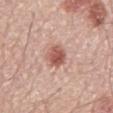Impression:
No biopsy was performed on this lesion — it was imaged during a full skin examination and was not determined to be concerning.
Clinical summary:
On the mid back. This is a white-light tile. The recorded lesion diameter is about 3 mm. Cropped from a whole-body photographic skin survey; the tile spans about 15 mm. A male subject roughly 70 years of age.